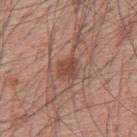– notes: imaged on a skin check; not biopsied
– subject: male, about 55 years old
– diameter: ~2.5 mm (longest diameter)
– image source: total-body-photography crop, ~15 mm field of view
– anatomic site: the mid back
– TBP lesion metrics: a lesion area of about 5 mm², a shape eccentricity near 0.5, and a shape-asymmetry score of about 0.3 (0 = symmetric); a lesion color around L≈46 a*≈23 b*≈27 in CIELAB, a lesion–skin lightness drop of about 9, and a normalized border contrast of about 7; a peripheral color-asymmetry measure near 0.5; a detector confidence of about 100 out of 100 that the crop contains a lesion
– lighting: white-light illumination